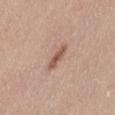Case summary:
• follow-up — no biopsy performed (imaged during a skin exam)
• anatomic site — the lower back
• lesion diameter — ≈3 mm
• illumination — white-light
• image source — ~15 mm crop, total-body skin-cancer survey
• subject — female, aged approximately 45